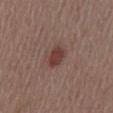Recorded during total-body skin imaging; not selected for excision or biopsy. A male subject, approximately 55 years of age. The lesion-visualizer software estimated a detector confidence of about 100 out of 100 that the crop contains a lesion. From the abdomen. This is a white-light tile. A 15 mm close-up extracted from a 3D total-body photography capture. The recorded lesion diameter is about 3 mm.